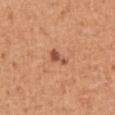The lesion was photographed on a routine skin check and not biopsied; there is no pathology result. This image is a 15 mm lesion crop taken from a total-body photograph. This is a white-light tile. On the front of the torso. A male patient, in their mid- to late 50s. The lesion's longest dimension is about 2.5 mm. The total-body-photography lesion software estimated border irregularity of about 4 on a 0–10 scale, a color-variation rating of about 1/10, and peripheral color asymmetry of about 0.